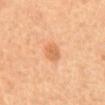Q: Was this lesion biopsied?
A: imaged on a skin check; not biopsied
Q: Where on the body is the lesion?
A: the back
Q: Who is the patient?
A: female
Q: How was this image acquired?
A: total-body-photography crop, ~15 mm field of view